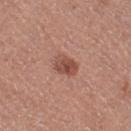site: right thigh
automated_metrics:
  cielab_L: 49
  cielab_a: 24
  cielab_b: 27
  vs_skin_darker_L: 11.0
  vs_skin_contrast_norm: 8.0
  border_irregularity_0_10: 1.0
  peripheral_color_asymmetry: 1.5
  nevus_likeness_0_100: 85
  lesion_detection_confidence_0_100: 100
lesion_size:
  long_diameter_mm_approx: 3.0
image:
  source: total-body photography crop
  field_of_view_mm: 15
patient:
  sex: female
  age_approx: 50
lighting: white-light